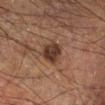notes: imaged on a skin check; not biopsied
site: the right lower leg
patient: male, in their 50s
acquisition: total-body-photography crop, ~15 mm field of view